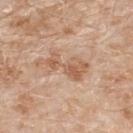Recorded during total-body skin imaging; not selected for excision or biopsy.
Located on the upper back.
A 15 mm crop from a total-body photograph taken for skin-cancer surveillance.
The subject is a male roughly 80 years of age.
Longest diameter approximately 6 mm.
Captured under white-light illumination.